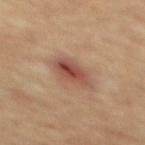  biopsy_status: not biopsied; imaged during a skin examination
  site: mid back
  lighting: cross-polarized
  patient:
    sex: male
    age_approx: 65
  lesion_size:
    long_diameter_mm_approx: 4.0
  automated_metrics:
    border_irregularity_0_10: 2.0
    color_variation_0_10: 6.5
    peripheral_color_asymmetry: 2.5
  image:
    source: total-body photography crop
    field_of_view_mm: 15